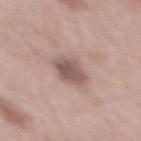Imaged during a routine full-body skin examination; the lesion was not biopsied and no histopathology is available. The lesion is located on the mid back. The tile uses white-light illumination. The recorded lesion diameter is about 4 mm. Cropped from a total-body skin-imaging series; the visible field is about 15 mm. The patient is a male aged 53 to 57. The lesion-visualizer software estimated a lesion color around L≈54 a*≈18 b*≈21 in CIELAB. And it measured a border-irregularity index near 2/10, a color-variation rating of about 3.5/10, and a peripheral color-asymmetry measure near 1.